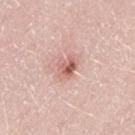On the leg. A male patient, aged 48–52. A region of skin cropped from a whole-body photographic capture, roughly 15 mm wide.